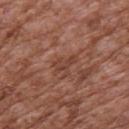Imaged during a routine full-body skin examination; the lesion was not biopsied and no histopathology is available.
About 3 mm across.
A male patient, approximately 75 years of age.
On the upper back.
Automated tile analysis of the lesion measured border irregularity of about 6.5 on a 0–10 scale, a within-lesion color-variation index near 1.5/10, and a peripheral color-asymmetry measure near 0.5. The analysis additionally found a classifier nevus-likeness of about 0/100 and a lesion-detection confidence of about 100/100.
Cropped from a whole-body photographic skin survey; the tile spans about 15 mm.